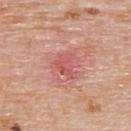• workup · imaged on a skin check; not biopsied
• lesion size · ≈3.5 mm
• image · ~15 mm tile from a whole-body skin photo
• anatomic site · the upper back
• patient · male, approximately 75 years of age
• tile lighting · white-light